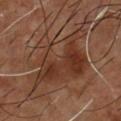Recorded during total-body skin imaging; not selected for excision or biopsy. A region of skin cropped from a whole-body photographic capture, roughly 15 mm wide. The lesion is on the front of the torso. A male patient, aged 53–57. The tile uses cross-polarized illumination.